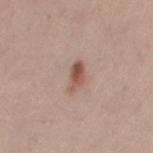biopsy_status: not biopsied; imaged during a skin examination
site: left thigh
image:
  source: total-body photography crop
  field_of_view_mm: 15
lighting: white-light
patient:
  sex: female
  age_approx: 25
lesion_size:
  long_diameter_mm_approx: 3.5